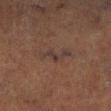The lesion was photographed on a routine skin check and not biopsied; there is no pathology result.
From the left lower leg.
Approximately 4 mm at its widest.
A 15 mm crop from a total-body photograph taken for skin-cancer surveillance.
A male patient aged 73–77.